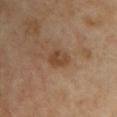The lesion was tiled from a total-body skin photograph and was not biopsied.
The lesion is on the chest.
The total-body-photography lesion software estimated an area of roughly 5 mm², an outline eccentricity of about 0.65 (0 = round, 1 = elongated), and a shape-asymmetry score of about 0.3 (0 = symmetric).
A region of skin cropped from a whole-body photographic capture, roughly 15 mm wide.
The recorded lesion diameter is about 3 mm.
The subject is a female aged 38–42.